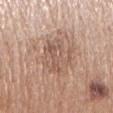Case summary:
• workup — imaged on a skin check; not biopsied
• diameter — ≈5.5 mm
• image-analysis metrics — a mean CIELAB color near L≈56 a*≈18 b*≈27 and about 8 CIELAB-L* units darker than the surrounding skin; a nevus-likeness score of about 0/100 and a detector confidence of about 100 out of 100 that the crop contains a lesion
• image — ~15 mm tile from a whole-body skin photo
• anatomic site — the arm
• subject — female, approximately 65 years of age
• tile lighting — white-light illumination